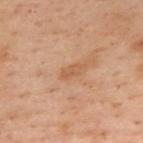  biopsy_status: not biopsied; imaged during a skin examination
  image:
    source: total-body photography crop
    field_of_view_mm: 15
  patient:
    sex: female
    age_approx: 55
  site: upper back